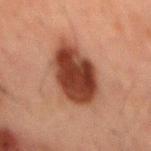  automated_metrics:
    area_mm2_approx: 22.0
    eccentricity: 0.85
    color_variation_0_10: 4.5
    peripheral_color_asymmetry: 1.5
    nevus_likeness_0_100: 100
    lesion_detection_confidence_0_100: 100
  patient:
    sex: male
    age_approx: 50
  lighting: cross-polarized
  image:
    source: total-body photography crop
    field_of_view_mm: 15
  site: mid back